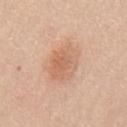Part of a total-body skin-imaging series; this lesion was reviewed on a skin check and was not flagged for biopsy. A male subject, roughly 35 years of age. On the back. This is a white-light tile. A lesion tile, about 15 mm wide, cut from a 3D total-body photograph. Automated image analysis of the tile measured an outline eccentricity of about 0.6 (0 = round, 1 = elongated) and a symmetry-axis asymmetry near 0.15. And it measured a mean CIELAB color near L≈64 a*≈21 b*≈33 and a lesion-to-skin contrast of about 5.5 (normalized; higher = more distinct). It also reported a border-irregularity rating of about 2/10, internal color variation of about 4 on a 0–10 scale, and radial color variation of about 1. The analysis additionally found a detector confidence of about 100 out of 100 that the crop contains a lesion. About 5 mm across.